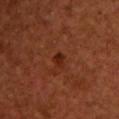biopsy_status: not biopsied; imaged during a skin examination
image:
  source: total-body photography crop
  field_of_view_mm: 15
automated_metrics:
  shape_asymmetry: 0.25
  border_irregularity_0_10: 2.0
  color_variation_0_10: 1.0
  peripheral_color_asymmetry: 0.0
  nevus_likeness_0_100: 85
  lesion_detection_confidence_0_100: 100
site: left upper arm
lighting: cross-polarized
patient:
  sex: male
  age_approx: 45